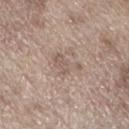notes = imaged on a skin check; not biopsied
imaging modality = 15 mm crop, total-body photography
lighting = white-light illumination
subject = male, roughly 70 years of age
lesion diameter = ~4 mm (longest diameter)
location = the left lower leg